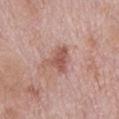Q: Is there a histopathology result?
A: imaged on a skin check; not biopsied
Q: What is the lesion's diameter?
A: ~4 mm (longest diameter)
Q: Where on the body is the lesion?
A: the mid back
Q: What are the patient's age and sex?
A: male, aged 73–77
Q: What is the imaging modality?
A: ~15 mm crop, total-body skin-cancer survey
Q: Automated lesion metrics?
A: an area of roughly 6 mm², a shape eccentricity near 0.85, and a shape-asymmetry score of about 0.45 (0 = symmetric); a lesion color around L≈54 a*≈24 b*≈25 in CIELAB and a lesion-to-skin contrast of about 7.5 (normalized; higher = more distinct)
Q: How was the tile lit?
A: white-light